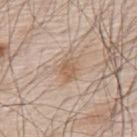{
  "site": "upper back",
  "lighting": "white-light",
  "lesion_size": {
    "long_diameter_mm_approx": 3.5
  },
  "automated_metrics": {
    "area_mm2_approx": 6.0,
    "eccentricity": 0.7,
    "shape_asymmetry": 0.25,
    "cielab_L": 60,
    "cielab_a": 16,
    "cielab_b": 30,
    "lesion_detection_confidence_0_100": 100
  },
  "patient": {
    "sex": "male",
    "age_approx": 80
  },
  "image": {
    "source": "total-body photography crop",
    "field_of_view_mm": 15
  }
}